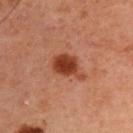Imaged during a routine full-body skin examination; the lesion was not biopsied and no histopathology is available.
A male subject, approximately 60 years of age.
The total-body-photography lesion software estimated a classifier nevus-likeness of about 100/100 and lesion-presence confidence of about 100/100.
From the left upper arm.
Approximately 4 mm at its widest.
This is a cross-polarized tile.
A 15 mm close-up tile from a total-body photography series done for melanoma screening.